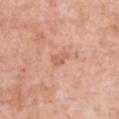The lesion was tiled from a total-body skin photograph and was not biopsied.
A close-up tile cropped from a whole-body skin photograph, about 15 mm across.
A female subject about 50 years old.
Longest diameter approximately 2.5 mm.
The tile uses white-light illumination.
The lesion is located on the front of the torso.